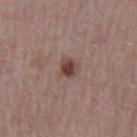Q: Was this lesion biopsied?
A: imaged on a skin check; not biopsied
Q: Where on the body is the lesion?
A: the mid back
Q: What lighting was used for the tile?
A: white-light illumination
Q: What is the imaging modality?
A: 15 mm crop, total-body photography
Q: Who is the patient?
A: male, aged around 75